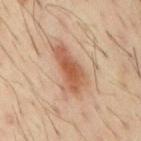Impression:
This lesion was catalogued during total-body skin photography and was not selected for biopsy.
Background:
A 15 mm close-up tile from a total-body photography series done for melanoma screening. The subject is a male aged 58 to 62. Captured under cross-polarized illumination. On the mid back.